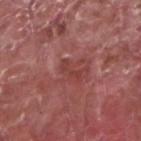The lesion was tiled from a total-body skin photograph and was not biopsied. Approximately 3.5 mm at its widest. From the left forearm. An algorithmic analysis of the crop reported a within-lesion color-variation index near 0/10 and peripheral color asymmetry of about 0. It also reported an automated nevus-likeness rating near 0 out of 100 and a lesion-detection confidence of about 100/100. A male patient aged around 40. A roughly 15 mm field-of-view crop from a total-body skin photograph. Imaged with white-light lighting.